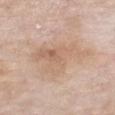Clinical impression:
This lesion was catalogued during total-body skin photography and was not selected for biopsy.
Background:
This is a white-light tile. The lesion is located on the chest. The recorded lesion diameter is about 7.5 mm. A female subject, aged around 75. Cropped from a total-body skin-imaging series; the visible field is about 15 mm.